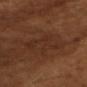Located on the head or neck. The total-body-photography lesion software estimated a footprint of about 12 mm² and a shape eccentricity near 0.8. It also reported a border-irregularity rating of about 9.5/10, a within-lesion color-variation index near 2/10, and radial color variation of about 0.5. A region of skin cropped from a whole-body photographic capture, roughly 15 mm wide. The recorded lesion diameter is about 5.5 mm. Captured under cross-polarized illumination. The patient is a male approximately 60 years of age.